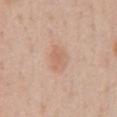A male subject, aged around 55. This is a white-light tile. A close-up tile cropped from a whole-body skin photograph, about 15 mm across. The lesion is located on the abdomen.